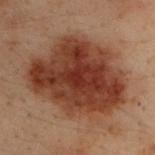Imaged during a routine full-body skin examination; the lesion was not biopsied and no histopathology is available. A close-up tile cropped from a whole-body skin photograph, about 15 mm across. A male patient, about 30 years old. From the upper back. The recorded lesion diameter is about 10.5 mm.